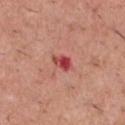- workup — imaged on a skin check; not biopsied
- patient — male, aged around 45
- body site — the front of the torso
- acquisition — ~15 mm tile from a whole-body skin photo